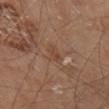| key | value |
|---|---|
| notes | total-body-photography surveillance lesion; no biopsy |
| anatomic site | the leg |
| diameter | about 3 mm |
| patient | male, in their 70s |
| image | ~15 mm tile from a whole-body skin photo |